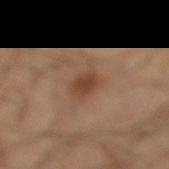Notes:
– workup — imaged on a skin check; not biopsied
– patient — male, in their 50s
– acquisition — 15 mm crop, total-body photography
– body site — the left leg
– lighting — cross-polarized illumination
– automated metrics — an area of roughly 5 mm², a shape eccentricity near 0.7, and a symmetry-axis asymmetry near 0.25; a lesion color around L≈42 a*≈19 b*≈29 in CIELAB, a lesion–skin lightness drop of about 9, and a normalized border contrast of about 7.5; an automated nevus-likeness rating near 85 out of 100 and lesion-presence confidence of about 100/100
– size — ~3 mm (longest diameter)